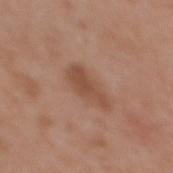Captured during whole-body skin photography for melanoma surveillance; the lesion was not biopsied.
The lesion is on the back.
The lesion's longest dimension is about 5 mm.
The total-body-photography lesion software estimated an area of roughly 8 mm², a shape eccentricity near 0.9, and a shape-asymmetry score of about 0.25 (0 = symmetric). And it measured a mean CIELAB color near L≈49 a*≈21 b*≈30, about 8 CIELAB-L* units darker than the surrounding skin, and a normalized border contrast of about 6.5. And it measured a border-irregularity index near 3.5/10 and a peripheral color-asymmetry measure near 1. It also reported a lesion-detection confidence of about 100/100.
Cropped from a whole-body photographic skin survey; the tile spans about 15 mm.
The subject is a female aged 33–37.
Imaged with white-light lighting.